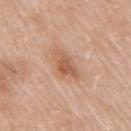{
  "biopsy_status": "not biopsied; imaged during a skin examination",
  "automated_metrics": {
    "border_irregularity_0_10": 4.0,
    "color_variation_0_10": 3.5,
    "peripheral_color_asymmetry": 1.0
  },
  "site": "right upper arm",
  "lighting": "white-light",
  "image": {
    "source": "total-body photography crop",
    "field_of_view_mm": 15
  },
  "patient": {
    "sex": "female",
    "age_approx": 55
  },
  "lesion_size": {
    "long_diameter_mm_approx": 4.0
  }
}